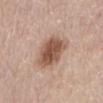No biopsy was performed on this lesion — it was imaged during a full skin examination and was not determined to be concerning. On the abdomen. A close-up tile cropped from a whole-body skin photograph, about 15 mm across. A female subject approximately 65 years of age.